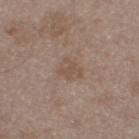Recorded during total-body skin imaging; not selected for excision or biopsy.